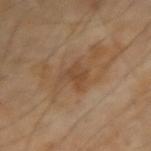Impression:
The lesion was photographed on a routine skin check and not biopsied; there is no pathology result.
Image and clinical context:
This image is a 15 mm lesion crop taken from a total-body photograph. The lesion is located on the right forearm.